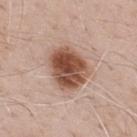<record>
<biopsy_status>not biopsied; imaged during a skin examination</biopsy_status>
<lighting>white-light</lighting>
<patient>
  <sex>male</sex>
  <age_approx>70</age_approx>
</patient>
<site>upper back</site>
<image>
  <source>total-body photography crop</source>
  <field_of_view_mm>15</field_of_view_mm>
</image>
<lesion_size>
  <long_diameter_mm_approx>5.0</long_diameter_mm_approx>
</lesion_size>
</record>